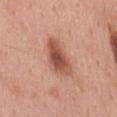{
  "biopsy_status": "not biopsied; imaged during a skin examination",
  "site": "mid back",
  "patient": {
    "sex": "male",
    "age_approx": 60
  },
  "image": {
    "source": "total-body photography crop",
    "field_of_view_mm": 15
  }
}